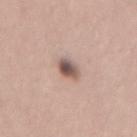biopsy status = imaged on a skin check; not biopsied | lighting = white-light | imaging modality = total-body-photography crop, ~15 mm field of view | location = the mid back | subject = female, in their mid- to late 20s.